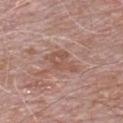The lesion was photographed on a routine skin check and not biopsied; there is no pathology result.
Cropped from a whole-body photographic skin survey; the tile spans about 15 mm.
About 4 mm across.
A male subject, aged around 70.
Imaged with white-light lighting.
From the chest.